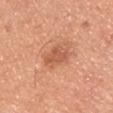follow-up: no biopsy performed (imaged during a skin exam); location: the chest; patient: male, aged 43–47; image source: ~15 mm tile from a whole-body skin photo; illumination: white-light.